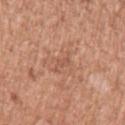Notes:
• subject: male, aged 43 to 47
• size: ≈3.5 mm
• anatomic site: the right upper arm
• imaging modality: total-body-photography crop, ~15 mm field of view
• lighting: white-light illumination